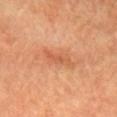Part of a total-body skin-imaging series; this lesion was reviewed on a skin check and was not flagged for biopsy.
The subject is a female roughly 75 years of age.
From the head or neck.
This image is a 15 mm lesion crop taken from a total-body photograph.